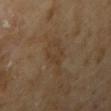<lesion>
  <biopsy_status>not biopsied; imaged during a skin examination</biopsy_status>
  <automated_metrics>
    <cielab_L>37</cielab_L>
    <cielab_a>14</cielab_a>
    <cielab_b>28</cielab_b>
    <vs_skin_darker_L>5.0</vs_skin_darker_L>
    <vs_skin_contrast_norm>5.0</vs_skin_contrast_norm>
    <color_variation_0_10>2.5</color_variation_0_10>
    <nevus_likeness_0_100>0</nevus_likeness_0_100>
    <lesion_detection_confidence_0_100>90</lesion_detection_confidence_0_100>
  </automated_metrics>
  <image>
    <source>total-body photography crop</source>
    <field_of_view_mm>15</field_of_view_mm>
  </image>
  <lighting>cross-polarized</lighting>
  <patient>
    <sex>female</sex>
    <age_approx>60</age_approx>
  </patient>
  <lesion_size>
    <long_diameter_mm_approx>5.0</long_diameter_mm_approx>
  </lesion_size>
  <site>right upper arm</site>
</lesion>